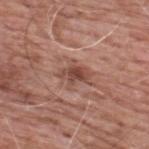Part of a total-body skin-imaging series; this lesion was reviewed on a skin check and was not flagged for biopsy.
The subject is a male approximately 60 years of age.
The lesion's longest dimension is about 3 mm.
The lesion is located on the upper back.
A region of skin cropped from a whole-body photographic capture, roughly 15 mm wide.